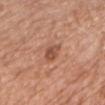notes: catalogued during a skin exam; not biopsied
patient: male, aged approximately 80
diameter: ~2.5 mm (longest diameter)
automated lesion analysis: a mean CIELAB color near L≈51 a*≈24 b*≈31 and roughly 10 lightness units darker than nearby skin
tile lighting: white-light
site: the chest
image: 15 mm crop, total-body photography